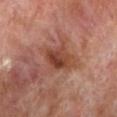No biopsy was performed on this lesion — it was imaged during a full skin examination and was not determined to be concerning. A male patient in their 70s. The recorded lesion diameter is about 4 mm. The lesion is located on the left lower leg. A 15 mm close-up extracted from a 3D total-body photography capture. The lesion-visualizer software estimated a lesion area of about 8.5 mm² and an eccentricity of roughly 0.75. It also reported an automated nevus-likeness rating near 0 out of 100 and a detector confidence of about 100 out of 100 that the crop contains a lesion. The tile uses cross-polarized illumination.